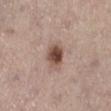Q: Was a biopsy performed?
A: no biopsy performed (imaged during a skin exam)
Q: How was this image acquired?
A: 15 mm crop, total-body photography
Q: Illumination type?
A: white-light
Q: What did automated image analysis measure?
A: a border-irregularity index near 2/10, internal color variation of about 6 on a 0–10 scale, and peripheral color asymmetry of about 2; a classifier nevus-likeness of about 95/100 and a lesion-detection confidence of about 100/100
Q: What are the patient's age and sex?
A: female, aged 63 to 67
Q: Lesion location?
A: the right lower leg
Q: Lesion size?
A: ≈3 mm